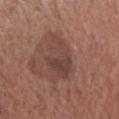Clinical impression: The lesion was photographed on a routine skin check and not biopsied; there is no pathology result. Acquisition and patient details: From the left forearm. Automated image analysis of the tile measured a nevus-likeness score of about 5/100 and a detector confidence of about 100 out of 100 that the crop contains a lesion. A female subject, aged 73 to 77. The recorded lesion diameter is about 5 mm. Cropped from a whole-body photographic skin survey; the tile spans about 15 mm.